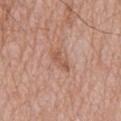Recorded during total-body skin imaging; not selected for excision or biopsy.
The lesion-visualizer software estimated a lesion–skin lightness drop of about 9 and a lesion-to-skin contrast of about 6.5 (normalized; higher = more distinct).
The tile uses white-light illumination.
The recorded lesion diameter is about 3 mm.
A roughly 15 mm field-of-view crop from a total-body skin photograph.
A male subject aged approximately 80.
The lesion is on the mid back.